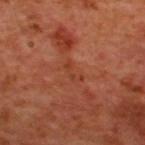Part of a total-body skin-imaging series; this lesion was reviewed on a skin check and was not flagged for biopsy. The lesion is located on the back. The tile uses cross-polarized illumination. The patient is a male in their 50s. A lesion tile, about 15 mm wide, cut from a 3D total-body photograph. About 2.5 mm across.